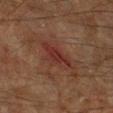Acquisition and patient details: The lesion is located on the right lower leg. A roughly 15 mm field-of-view crop from a total-body skin photograph. Captured under cross-polarized illumination. Approximately 5 mm at its widest. A male patient aged 58 to 62.